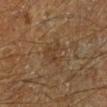Q: How was this image acquired?
A: ~15 mm tile from a whole-body skin photo
Q: How large is the lesion?
A: ≈3 mm
Q: What is the anatomic site?
A: the leg
Q: What are the patient's age and sex?
A: male, aged 63–67